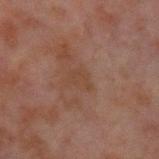follow-up: imaged on a skin check; not biopsied
subject: male, approximately 30 years of age
site: the left upper arm
image: ~15 mm crop, total-body skin-cancer survey
lesion diameter: ≈2.5 mm
tile lighting: cross-polarized illumination